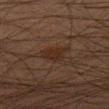Imaged during a routine full-body skin examination; the lesion was not biopsied and no histopathology is available. Automated image analysis of the tile measured a normalized lesion–skin contrast near 6.5. On the left thigh. A male patient aged approximately 35. The lesion's longest dimension is about 2.5 mm. A roughly 15 mm field-of-view crop from a total-body skin photograph. Imaged with cross-polarized lighting.